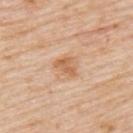{
  "biopsy_status": "not biopsied; imaged during a skin examination",
  "lesion_size": {
    "long_diameter_mm_approx": 2.5
  },
  "site": "left upper arm",
  "lighting": "white-light",
  "image": {
    "source": "total-body photography crop",
    "field_of_view_mm": 15
  },
  "patient": {
    "sex": "male",
    "age_approx": 75
  }
}